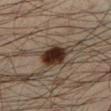Assessment:
The lesion was photographed on a routine skin check and not biopsied; there is no pathology result.